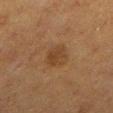| field | value |
|---|---|
| image source | ~15 mm tile from a whole-body skin photo |
| tile lighting | cross-polarized |
| lesion size | ≈3 mm |
| TBP lesion metrics | a mean CIELAB color near L≈33 a*≈16 b*≈29, about 6 CIELAB-L* units darker than the surrounding skin, and a lesion-to-skin contrast of about 6.5 (normalized; higher = more distinct) |
| site | the leg |
| patient | female, roughly 40 years of age |